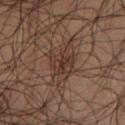<record>
<biopsy_status>not biopsied; imaged during a skin examination</biopsy_status>
<site>right thigh</site>
<image>
  <source>total-body photography crop</source>
  <field_of_view_mm>15</field_of_view_mm>
</image>
<lesion_size>
  <long_diameter_mm_approx>5.0</long_diameter_mm_approx>
</lesion_size>
<lighting>cross-polarized</lighting>
<patient>
  <sex>male</sex>
  <age_approx>50</age_approx>
</patient>
</record>